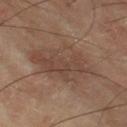notes = imaged on a skin check; not biopsied | diameter = about 8 mm | body site = the leg | patient = male, aged 63–67 | image-analysis metrics = an automated nevus-likeness rating near 0 out of 100 and lesion-presence confidence of about 100/100 | acquisition = ~15 mm crop, total-body skin-cancer survey | tile lighting = cross-polarized.